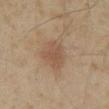The lesion was photographed on a routine skin check and not biopsied; there is no pathology result. The lesion's longest dimension is about 4 mm. A male subject, about 60 years old. Located on the front of the torso. Captured under cross-polarized illumination. Cropped from a whole-body photographic skin survey; the tile spans about 15 mm.